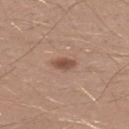biopsy_status: not biopsied; imaged during a skin examination
patient:
  sex: male
  age_approx: 30
lighting: white-light
image:
  source: total-body photography crop
  field_of_view_mm: 15
automated_metrics:
  color_variation_0_10: 2.0
  peripheral_color_asymmetry: 0.5
site: left lower leg
lesion_size:
  long_diameter_mm_approx: 3.0